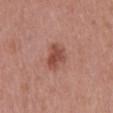workup=total-body-photography surveillance lesion; no biopsy | patient=male, aged 68–72 | imaging modality=~15 mm tile from a whole-body skin photo | TBP lesion metrics=a footprint of about 7.5 mm² and two-axis asymmetry of about 0.25; a lesion color around L≈49 a*≈25 b*≈28 in CIELAB, a lesion–skin lightness drop of about 10, and a normalized lesion–skin contrast near 7.5 | lighting=white-light illumination | anatomic site=the mid back | diameter=≈4 mm.